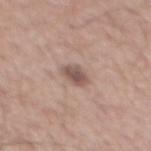Part of a total-body skin-imaging series; this lesion was reviewed on a skin check and was not flagged for biopsy.
On the mid back.
Automated image analysis of the tile measured a shape-asymmetry score of about 0.15 (0 = symmetric). The software also gave roughly 12 lightness units darker than nearby skin and a normalized lesion–skin contrast near 8. The software also gave border irregularity of about 2 on a 0–10 scale, a color-variation rating of about 3.5/10, and a peripheral color-asymmetry measure near 1. The analysis additionally found an automated nevus-likeness rating near 55 out of 100 and a lesion-detection confidence of about 100/100.
A male subject aged 53–57.
A roughly 15 mm field-of-view crop from a total-body skin photograph.